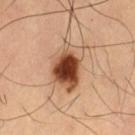Impression:
Recorded during total-body skin imaging; not selected for excision or biopsy.
Background:
This is a cross-polarized tile. The lesion's longest dimension is about 5.5 mm. The subject is a male approximately 60 years of age. The total-body-photography lesion software estimated an outline eccentricity of about 0.75 (0 = round, 1 = elongated) and a shape-asymmetry score of about 0.2 (0 = symmetric). The software also gave an automated nevus-likeness rating near 100 out of 100 and a lesion-detection confidence of about 100/100. The lesion is located on the left thigh. A 15 mm crop from a total-body photograph taken for skin-cancer surveillance.